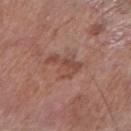notes: imaged on a skin check; not biopsied
TBP lesion metrics: a shape eccentricity near 0.9 and a symmetry-axis asymmetry near 0.55; roughly 8 lightness units darker than nearby skin
acquisition: ~15 mm tile from a whole-body skin photo
illumination: white-light
size: about 4 mm
subject: male, aged 58–62
body site: the arm